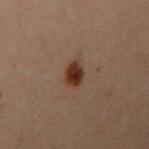| feature | finding |
|---|---|
| follow-up | total-body-photography surveillance lesion; no biopsy |
| patient | female, about 30 years old |
| image | total-body-photography crop, ~15 mm field of view |
| lighting | cross-polarized |
| location | the left arm |